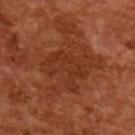Recorded during total-body skin imaging; not selected for excision or biopsy. The tile uses cross-polarized illumination. The subject is a male aged around 65. Automated tile analysis of the lesion measured an outline eccentricity of about 0.65 (0 = round, 1 = elongated) and two-axis asymmetry of about 0.45. The software also gave a border-irregularity index near 7/10 and peripheral color asymmetry of about 1. The recorded lesion diameter is about 5.5 mm. Cropped from a total-body skin-imaging series; the visible field is about 15 mm.